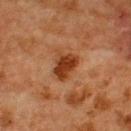Assessment:
Recorded during total-body skin imaging; not selected for excision or biopsy.